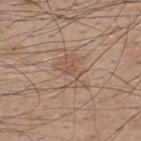Captured during whole-body skin photography for melanoma surveillance; the lesion was not biopsied.
A close-up tile cropped from a whole-body skin photograph, about 15 mm across.
A male patient aged 48 to 52.
On the upper back.